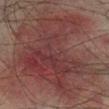A lesion tile, about 15 mm wide, cut from a 3D total-body photograph.
The lesion-visualizer software estimated a classifier nevus-likeness of about 5/100 and lesion-presence confidence of about 90/100.
This is a cross-polarized tile.
From the right lower leg.
The lesion's longest dimension is about 13 mm.
The subject is a male roughly 75 years of age.